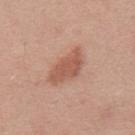Q: Is there a histopathology result?
A: catalogued during a skin exam; not biopsied
Q: How was the tile lit?
A: white-light
Q: Lesion size?
A: ~5 mm (longest diameter)
Q: What is the imaging modality?
A: ~15 mm crop, total-body skin-cancer survey
Q: What is the anatomic site?
A: the mid back
Q: Who is the patient?
A: male, aged 28–32